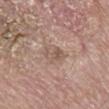workup — no biopsy performed (imaged during a skin exam)
patient — male, aged around 80
acquisition — total-body-photography crop, ~15 mm field of view
body site — the mid back